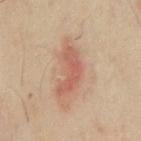Notes:
• follow-up: imaged on a skin check; not biopsied
• tile lighting: cross-polarized
• patient: male, in their mid- to late 60s
• image: ~15 mm crop, total-body skin-cancer survey
• image-analysis metrics: a footprint of about 11 mm² and an eccentricity of roughly 0.9; a color-variation rating of about 3/10 and peripheral color asymmetry of about 1
• body site: the chest